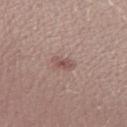notes: total-body-photography surveillance lesion; no biopsy
subject: female, aged 33 to 37
image: ~15 mm crop, total-body skin-cancer survey
location: the left forearm
lesion size: ~2.5 mm (longest diameter)
automated metrics: internal color variation of about 3 on a 0–10 scale and peripheral color asymmetry of about 1
tile lighting: white-light illumination